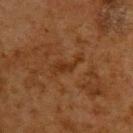Case summary:
- workup — no biopsy performed (imaged during a skin exam)
- anatomic site — the upper back
- automated metrics — an area of roughly 3.5 mm², an eccentricity of roughly 0.95, and a shape-asymmetry score of about 0.65 (0 = symmetric); a lesion color around L≈27 a*≈20 b*≈30 in CIELAB, about 6 CIELAB-L* units darker than the surrounding skin, and a normalized border contrast of about 6.5; an automated nevus-likeness rating near 0 out of 100
- diameter — ~4 mm (longest diameter)
- acquisition — 15 mm crop, total-body photography
- subject — male, aged approximately 60
- lighting — cross-polarized illumination